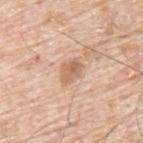| feature | finding |
|---|---|
| follow-up | no biopsy performed (imaged during a skin exam) |